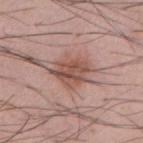{"biopsy_status": "not biopsied; imaged during a skin examination"}